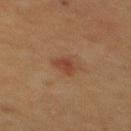Recorded during total-body skin imaging; not selected for excision or biopsy. Imaged with cross-polarized lighting. A male patient, about 50 years old. The lesion is located on the mid back. Cropped from a total-body skin-imaging series; the visible field is about 15 mm. An algorithmic analysis of the crop reported a lesion color around L≈33 a*≈19 b*≈26 in CIELAB and a lesion–skin lightness drop of about 7. The analysis additionally found a border-irregularity rating of about 4/10, internal color variation of about 2 on a 0–10 scale, and peripheral color asymmetry of about 0.5.